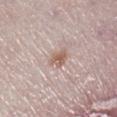<record>
<biopsy_status>not biopsied; imaged during a skin examination</biopsy_status>
<lighting>white-light</lighting>
<patient>
  <sex>female</sex>
  <age_approx>60</age_approx>
</patient>
<site>right lower leg</site>
<lesion_size>
  <long_diameter_mm_approx>2.5</long_diameter_mm_approx>
</lesion_size>
<image>
  <source>total-body photography crop</source>
  <field_of_view_mm>15</field_of_view_mm>
</image>
</record>